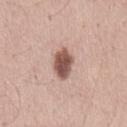Q: Was a biopsy performed?
A: no biopsy performed (imaged during a skin exam)
Q: How large is the lesion?
A: ~4 mm (longest diameter)
Q: What did automated image analysis measure?
A: a lesion color around L≈53 a*≈20 b*≈24 in CIELAB and a lesion-to-skin contrast of about 11 (normalized; higher = more distinct)
Q: Patient demographics?
A: male, aged approximately 35
Q: How was the tile lit?
A: white-light illumination
Q: What kind of image is this?
A: total-body-photography crop, ~15 mm field of view
Q: What is the anatomic site?
A: the mid back